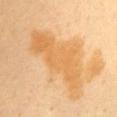notes: imaged on a skin check; not biopsied | image source: ~15 mm tile from a whole-body skin photo | lighting: cross-polarized | image-analysis metrics: border irregularity of about 6 on a 0–10 scale and a peripheral color-asymmetry measure near 1; a nevus-likeness score of about 0/100 | site: the chest | patient: female, aged 48 to 52 | diameter: ≈10.5 mm.